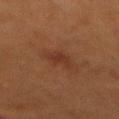workup = no biopsy performed (imaged during a skin exam)
automated lesion analysis = an automated nevus-likeness rating near 0 out of 100 and a lesion-detection confidence of about 100/100
subject = female, roughly 50 years of age
lesion size = ~3 mm (longest diameter)
image = total-body-photography crop, ~15 mm field of view
location = the back